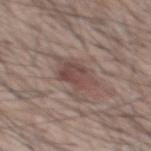Assessment:
The lesion was photographed on a routine skin check and not biopsied; there is no pathology result.
Context:
A male subject, about 60 years old. Located on the mid back. Cropped from a total-body skin-imaging series; the visible field is about 15 mm.